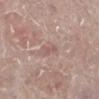Background:
The subject is a female about 70 years old. Imaged with white-light lighting. A roughly 15 mm field-of-view crop from a total-body skin photograph. From the right lower leg. The recorded lesion diameter is about 2.5 mm.